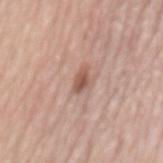Captured during whole-body skin photography for melanoma surveillance; the lesion was not biopsied. The lesion is on the mid back. Measured at roughly 3 mm in maximum diameter. Cropped from a total-body skin-imaging series; the visible field is about 15 mm. Captured under white-light illumination. A male patient, in their mid- to late 60s.